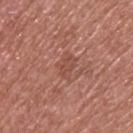{
  "biopsy_status": "not biopsied; imaged during a skin examination",
  "image": {
    "source": "total-body photography crop",
    "field_of_view_mm": 15
  },
  "site": "upper back",
  "patient": {
    "sex": "male",
    "age_approx": 55
  }
}